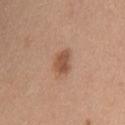Clinical impression:
Part of a total-body skin-imaging series; this lesion was reviewed on a skin check and was not flagged for biopsy.
Image and clinical context:
This is a white-light tile. A roughly 15 mm field-of-view crop from a total-body skin photograph. The lesion is located on the upper back. About 3 mm across. A female subject, in their 40s.